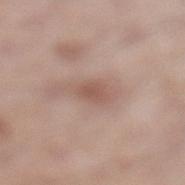Located on the left lower leg.
The subject is a male in their mid- to late 50s.
A region of skin cropped from a whole-body photographic capture, roughly 15 mm wide.